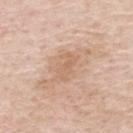{
  "biopsy_status": "not biopsied; imaged during a skin examination",
  "image": {
    "source": "total-body photography crop",
    "field_of_view_mm": 15
  },
  "automated_metrics": {
    "cielab_L": 64,
    "cielab_a": 18,
    "cielab_b": 32,
    "vs_skin_contrast_norm": 4.5,
    "nevus_likeness_0_100": 0,
    "lesion_detection_confidence_0_100": 100
  },
  "patient": {
    "sex": "male",
    "age_approx": 70
  },
  "lesion_size": {
    "long_diameter_mm_approx": 3.0
  },
  "site": "upper back"
}